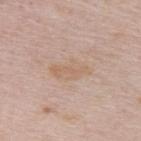Imaged during a routine full-body skin examination; the lesion was not biopsied and no histopathology is available. From the back. The tile uses white-light illumination. A roughly 15 mm field-of-view crop from a total-body skin photograph. A female subject, roughly 65 years of age. The lesion's longest dimension is about 4.5 mm. Automated image analysis of the tile measured a border-irregularity rating of about 3/10, a within-lesion color-variation index near 2/10, and a peripheral color-asymmetry measure near 0.5.